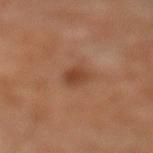From the left lower leg.
Longest diameter approximately 2.5 mm.
Captured under cross-polarized illumination.
The lesion-visualizer software estimated a shape eccentricity near 0.6. The software also gave a classifier nevus-likeness of about 35/100 and a detector confidence of about 100 out of 100 that the crop contains a lesion.
A male patient, aged 58–62.
Cropped from a total-body skin-imaging series; the visible field is about 15 mm.